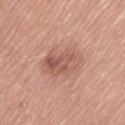The lesion was tiled from a total-body skin photograph and was not biopsied.
A 15 mm crop from a total-body photograph taken for skin-cancer surveillance.
A female subject about 65 years old.
Located on the right thigh.